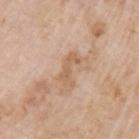Assessment: The lesion was photographed on a routine skin check and not biopsied; there is no pathology result. Image and clinical context: The subject is a male aged 58 to 62. Located on the left upper arm. A roughly 15 mm field-of-view crop from a total-body skin photograph.